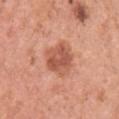Clinical impression:
The lesion was tiled from a total-body skin photograph and was not biopsied.
Clinical summary:
The patient is a female in their 50s. The lesion's longest dimension is about 4.5 mm. A 15 mm close-up extracted from a 3D total-body photography capture. On the left upper arm. Captured under white-light illumination.